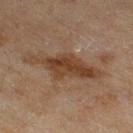The lesion was photographed on a routine skin check and not biopsied; there is no pathology result. Located on the right thigh. A close-up tile cropped from a whole-body skin photograph, about 15 mm across. Imaged with cross-polarized lighting. A female subject about 60 years old. About 8.5 mm across.